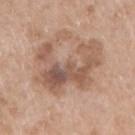{"biopsy_status": "not biopsied; imaged during a skin examination", "lesion_size": {"long_diameter_mm_approx": 8.0}, "image": {"source": "total-body photography crop", "field_of_view_mm": 15}, "automated_metrics": {"nevus_likeness_0_100": 10, "lesion_detection_confidence_0_100": 100}, "lighting": "white-light", "patient": {"sex": "female", "age_approx": 75}, "site": "right upper arm"}